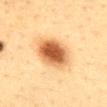{"biopsy_status": "not biopsied; imaged during a skin examination", "patient": {"sex": "female", "age_approx": 40}, "lesion_size": {"long_diameter_mm_approx": 5.0}, "image": {"source": "total-body photography crop", "field_of_view_mm": 15}, "lighting": "cross-polarized", "site": "mid back"}